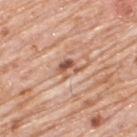A male patient, in their 80s. Automated tile analysis of the lesion measured an automated nevus-likeness rating near 0 out of 100 and a detector confidence of about 95 out of 100 that the crop contains a lesion. The lesion is on the upper back. The tile uses white-light illumination. Measured at roughly 3 mm in maximum diameter. A lesion tile, about 15 mm wide, cut from a 3D total-body photograph.